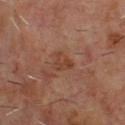Impression: Recorded during total-body skin imaging; not selected for excision or biopsy. Clinical summary: Automated image analysis of the tile measured an area of roughly 4 mm² and a shape eccentricity near 0.6. And it measured a border-irregularity rating of about 4/10, a within-lesion color-variation index near 2.5/10, and a peripheral color-asymmetry measure near 1. The analysis additionally found lesion-presence confidence of about 100/100. A male patient approximately 60 years of age. This is a cross-polarized tile. From the chest. Measured at roughly 3 mm in maximum diameter. A lesion tile, about 15 mm wide, cut from a 3D total-body photograph.